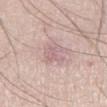site: right thigh
lighting: white-light
image:
  source: total-body photography crop
  field_of_view_mm: 15
patient:
  sex: male
  age_approx: 65
lesion_size:
  long_diameter_mm_approx: 3.0